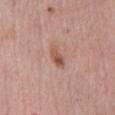Impression: Imaged during a routine full-body skin examination; the lesion was not biopsied and no histopathology is available. Image and clinical context: The lesion is located on the abdomen. A roughly 15 mm field-of-view crop from a total-body skin photograph. A female subject, in their mid-60s. The tile uses white-light illumination. The recorded lesion diameter is about 3 mm. Automated tile analysis of the lesion measured a border-irregularity index near 2.5/10, internal color variation of about 4.5 on a 0–10 scale, and peripheral color asymmetry of about 1.5. The software also gave a detector confidence of about 100 out of 100 that the crop contains a lesion.